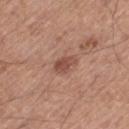Clinical impression: This lesion was catalogued during total-body skin photography and was not selected for biopsy. Context: A male patient, aged 63 to 67. Located on the leg. Automated tile analysis of the lesion measured a nevus-likeness score of about 40/100 and lesion-presence confidence of about 100/100. Imaged with white-light lighting. A lesion tile, about 15 mm wide, cut from a 3D total-body photograph. Longest diameter approximately 2.5 mm.